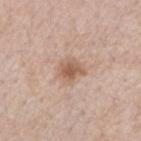biopsy_status: not biopsied; imaged during a skin examination
lesion_size:
  long_diameter_mm_approx: 3.0
image:
  source: total-body photography crop
  field_of_view_mm: 15
site: right forearm
automated_metrics:
  eccentricity: 0.55
  shape_asymmetry: 0.2
  cielab_L: 58
  cielab_a: 19
  cielab_b: 29
  vs_skin_darker_L: 10.0
  vs_skin_contrast_norm: 7.5
patient:
  sex: female
  age_approx: 40
lighting: white-light